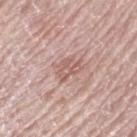acquisition = total-body-photography crop, ~15 mm field of view | location = the arm | TBP lesion metrics = an eccentricity of roughly 0.7; a border-irregularity index near 4/10, internal color variation of about 3 on a 0–10 scale, and a peripheral color-asymmetry measure near 1; a classifier nevus-likeness of about 0/100 and lesion-presence confidence of about 90/100 | patient = female, approximately 65 years of age | illumination = white-light illumination.